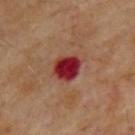Findings:
– imaging modality: ~15 mm crop, total-body skin-cancer survey
– diameter: about 3.5 mm
– location: the upper back
– image-analysis metrics: an average lesion color of about L≈33 a*≈36 b*≈26 (CIELAB), about 16 CIELAB-L* units darker than the surrounding skin, and a normalized border contrast of about 14
– subject: male, approximately 70 years of age
– illumination: cross-polarized illumination